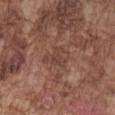No biopsy was performed on this lesion — it was imaged during a full skin examination and was not determined to be concerning.
The lesion is located on the chest.
A male patient in their mid-70s.
About 3 mm across.
The tile uses white-light illumination.
Cropped from a total-body skin-imaging series; the visible field is about 15 mm.
The total-body-photography lesion software estimated a footprint of about 6.5 mm², an eccentricity of roughly 0.55, and a shape-asymmetry score of about 0.35 (0 = symmetric). The software also gave an average lesion color of about L≈42 a*≈20 b*≈25 (CIELAB), a lesion–skin lightness drop of about 6, and a normalized border contrast of about 5. It also reported a within-lesion color-variation index near 3/10 and peripheral color asymmetry of about 1.